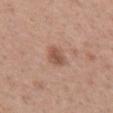{
  "biopsy_status": "not biopsied; imaged during a skin examination",
  "patient": {
    "sex": "female",
    "age_approx": 30
  },
  "lighting": "white-light",
  "image": {
    "source": "total-body photography crop",
    "field_of_view_mm": 15
  },
  "automated_metrics": {
    "eccentricity": 0.25,
    "shape_asymmetry": 0.25
  },
  "site": "left forearm",
  "lesion_size": {
    "long_diameter_mm_approx": 2.5
  }
}